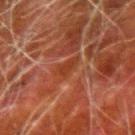Captured during whole-body skin photography for melanoma surveillance; the lesion was not biopsied. Captured under cross-polarized illumination. The recorded lesion diameter is about 3 mm. Located on the right forearm. The subject is a male in their 80s. A 15 mm crop from a total-body photograph taken for skin-cancer surveillance. The total-body-photography lesion software estimated a lesion area of about 4 mm², an eccentricity of roughly 0.75, and a symmetry-axis asymmetry near 0.35.